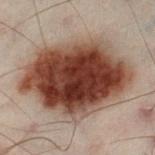Captured during whole-body skin photography for melanoma surveillance; the lesion was not biopsied. The subject is a male aged 48 to 52. Approximately 11.5 mm at its widest. Located on the left lower leg. A 15 mm crop from a total-body photograph taken for skin-cancer surveillance. This is a cross-polarized tile. Automated tile analysis of the lesion measured a lesion area of about 70 mm², a shape eccentricity near 0.7, and two-axis asymmetry of about 0.15. The software also gave a classifier nevus-likeness of about 100/100 and a detector confidence of about 100 out of 100 that the crop contains a lesion.